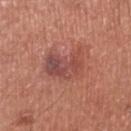Findings:
- lesion size: about 5 mm
- site: the leg
- subject: male, about 70 years old
- image source: total-body-photography crop, ~15 mm field of view
- TBP lesion metrics: about 9 CIELAB-L* units darker than the surrounding skin and a lesion-to-skin contrast of about 7 (normalized; higher = more distinct); border irregularity of about 4 on a 0–10 scale, internal color variation of about 8 on a 0–10 scale, and peripheral color asymmetry of about 2.5